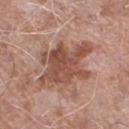workup = total-body-photography surveillance lesion; no biopsy | TBP lesion metrics = a lesion–skin lightness drop of about 12 and a normalized border contrast of about 8.5; a nevus-likeness score of about 0/100 and lesion-presence confidence of about 100/100 | anatomic site = the left lower leg | diameter = ~7.5 mm (longest diameter) | patient = male, aged around 75 | imaging modality = total-body-photography crop, ~15 mm field of view | lighting = white-light.